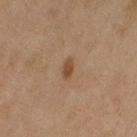Impression:
The lesion was tiled from a total-body skin photograph and was not biopsied.
Background:
The lesion's longest dimension is about 2.5 mm. On the left upper arm. Automated image analysis of the tile measured a lesion area of about 2.5 mm², an outline eccentricity of about 0.85 (0 = round, 1 = elongated), and two-axis asymmetry of about 0.25. It also reported a within-lesion color-variation index near 1/10 and radial color variation of about 0.5. A female patient, about 60 years old. The tile uses cross-polarized illumination. This image is a 15 mm lesion crop taken from a total-body photograph.